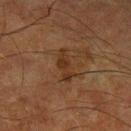| feature | finding |
|---|---|
| biopsy status | no biopsy performed (imaged during a skin exam) |
| lighting | cross-polarized illumination |
| site | the left lower leg |
| subject | male, roughly 65 years of age |
| image | total-body-photography crop, ~15 mm field of view |
| TBP lesion metrics | a lesion area of about 5.5 mm², a shape eccentricity near 0.9, and two-axis asymmetry of about 0.35; a border-irregularity index near 4.5/10 and a color-variation rating of about 1.5/10; a classifier nevus-likeness of about 5/100 and lesion-presence confidence of about 100/100 |